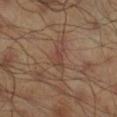{"biopsy_status": "not biopsied; imaged during a skin examination", "site": "right lower leg", "lighting": "cross-polarized", "image": {"source": "total-body photography crop", "field_of_view_mm": 15}, "patient": {"sex": "male", "age_approx": 45}, "automated_metrics": {"area_mm2_approx": 3.5, "eccentricity": 0.75, "shape_asymmetry": 0.4, "border_irregularity_0_10": 4.0, "peripheral_color_asymmetry": 0.5, "nevus_likeness_0_100": 0, "lesion_detection_confidence_0_100": 90}}